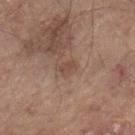Assessment:
No biopsy was performed on this lesion — it was imaged during a full skin examination and was not determined to be concerning.
Context:
A male patient, approximately 80 years of age. The tile uses white-light illumination. The lesion is on the right lower leg. Cropped from a total-body skin-imaging series; the visible field is about 15 mm. Automated tile analysis of the lesion measured a footprint of about 3.5 mm² and two-axis asymmetry of about 0.35. And it measured a border-irregularity rating of about 3/10, internal color variation of about 1 on a 0–10 scale, and peripheral color asymmetry of about 0.5.